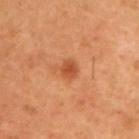Clinical impression:
The lesion was photographed on a routine skin check and not biopsied; there is no pathology result.
Clinical summary:
About 2 mm across. The lesion is located on the right upper arm. This image is a 15 mm lesion crop taken from a total-body photograph. A male subject aged approximately 60.